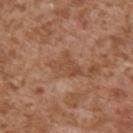Q: Lesion location?
A: the right upper arm
Q: What are the patient's age and sex?
A: male, aged 43–47
Q: What lighting was used for the tile?
A: white-light
Q: What kind of image is this?
A: total-body-photography crop, ~15 mm field of view
Q: How large is the lesion?
A: about 3.5 mm